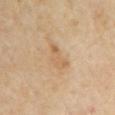follow-up = total-body-photography surveillance lesion; no biopsy | anatomic site = the left upper arm | lighting = cross-polarized | patient = female, aged around 60 | size = ~4 mm (longest diameter) | acquisition = total-body-photography crop, ~15 mm field of view.